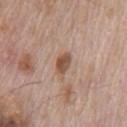Captured during whole-body skin photography for melanoma surveillance; the lesion was not biopsied.
Longest diameter approximately 3 mm.
Imaged with white-light lighting.
Cropped from a whole-body photographic skin survey; the tile spans about 15 mm.
On the chest.
A male patient, aged 68–72.